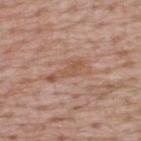Imaged during a routine full-body skin examination; the lesion was not biopsied and no histopathology is available. A 15 mm close-up tile from a total-body photography series done for melanoma screening. A male patient, aged 63 to 67. The lesion is on the upper back. The tile uses white-light illumination.